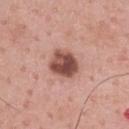notes: no biopsy performed (imaged during a skin exam)
tile lighting: white-light
patient: male, roughly 60 years of age
imaging modality: ~15 mm tile from a whole-body skin photo
lesion diameter: ~4 mm (longest diameter)
body site: the chest